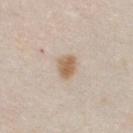follow-up=catalogued during a skin exam; not biopsied
subject=male, aged 33–37
imaging modality=15 mm crop, total-body photography
location=the chest